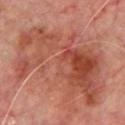The lesion was photographed on a routine skin check and not biopsied; there is no pathology result. A region of skin cropped from a whole-body photographic capture, roughly 15 mm wide. A male patient approximately 65 years of age. The lesion is located on the front of the torso. Longest diameter approximately 12 mm.